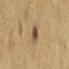Clinical impression: The lesion was photographed on a routine skin check and not biopsied; there is no pathology result. Clinical summary: The lesion-visualizer software estimated two-axis asymmetry of about 0.2. The software also gave a border-irregularity index near 2.5/10, a color-variation rating of about 4/10, and peripheral color asymmetry of about 1. And it measured a lesion-detection confidence of about 100/100. Captured under cross-polarized illumination. The recorded lesion diameter is about 3 mm. A 15 mm crop from a total-body photograph taken for skin-cancer surveillance. A female patient, approximately 55 years of age. The lesion is located on the mid back.